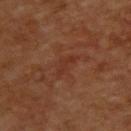Assessment:
Recorded during total-body skin imaging; not selected for excision or biopsy.
Clinical summary:
About 3.5 mm across. A male patient, aged around 60. Imaged with cross-polarized lighting. A 15 mm close-up tile from a total-body photography series done for melanoma screening. On the upper back.